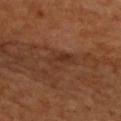Recorded during total-body skin imaging; not selected for excision or biopsy. The lesion is on the upper back. Cropped from a whole-body photographic skin survey; the tile spans about 15 mm. A male patient, aged 63 to 67.